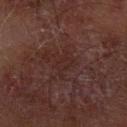The lesion was photographed on a routine skin check and not biopsied; there is no pathology result. The tile uses cross-polarized illumination. From the left forearm. The subject is a male aged approximately 70. A 15 mm crop from a total-body photograph taken for skin-cancer surveillance.